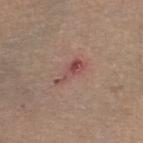This lesion was catalogued during total-body skin photography and was not selected for biopsy. A female subject approximately 60 years of age. A lesion tile, about 15 mm wide, cut from a 3D total-body photograph. From the left lower leg.